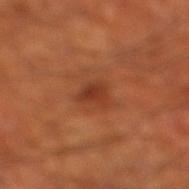Captured during whole-body skin photography for melanoma surveillance; the lesion was not biopsied.
The subject is a male aged 68 to 72.
This image is a 15 mm lesion crop taken from a total-body photograph.
The tile uses cross-polarized illumination.
On the right thigh.